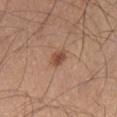Part of a total-body skin-imaging series; this lesion was reviewed on a skin check and was not flagged for biopsy. A male patient, in their mid-40s. Cropped from a whole-body photographic skin survey; the tile spans about 15 mm. Approximately 2.5 mm at its widest. Automated image analysis of the tile measured an area of roughly 4 mm², a shape eccentricity near 0.75, and a shape-asymmetry score of about 0.2 (0 = symmetric). And it measured a mean CIELAB color near L≈49 a*≈21 b*≈30, about 10 CIELAB-L* units darker than the surrounding skin, and a normalized lesion–skin contrast near 7.5. The software also gave a nevus-likeness score of about 90/100 and lesion-presence confidence of about 100/100. The lesion is on the right lower leg.